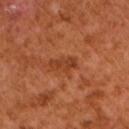Impression: Captured during whole-body skin photography for melanoma surveillance; the lesion was not biopsied. Clinical summary: Automated image analysis of the tile measured a lesion area of about 4 mm², an outline eccentricity of about 0.9 (0 = round, 1 = elongated), and two-axis asymmetry of about 0.4. The software also gave a border-irregularity rating of about 5/10, a within-lesion color-variation index near 0.5/10, and peripheral color asymmetry of about 0. The software also gave a classifier nevus-likeness of about 5/100 and a detector confidence of about 100 out of 100 that the crop contains a lesion. A roughly 15 mm field-of-view crop from a total-body skin photograph. Approximately 3.5 mm at its widest. The patient is a male aged approximately 65.